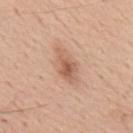Case summary:
• notes · total-body-photography surveillance lesion; no biopsy
• body site · the upper back
• image · ~15 mm crop, total-body skin-cancer survey
• lesion diameter · ≈4 mm
• patient · male, aged around 55
• illumination · white-light illumination
• automated lesion analysis · an area of roughly 7 mm², an outline eccentricity of about 0.85 (0 = round, 1 = elongated), and two-axis asymmetry of about 0.3; a border-irregularity rating of about 4/10, internal color variation of about 4.5 on a 0–10 scale, and a peripheral color-asymmetry measure near 1